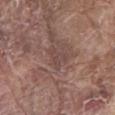follow-up = total-body-photography surveillance lesion; no biopsy | subject = male, in their 80s | size = about 3 mm | anatomic site = the mid back | imaging modality = ~15 mm tile from a whole-body skin photo.